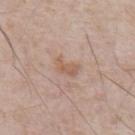Recorded during total-body skin imaging; not selected for excision or biopsy. Located on the abdomen. A male subject roughly 80 years of age. Approximately 3 mm at its widest. This is a white-light tile. A 15 mm close-up extracted from a 3D total-body photography capture.